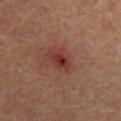{"biopsy_status": "not biopsied; imaged during a skin examination", "site": "mid back", "image": {"source": "total-body photography crop", "field_of_view_mm": 15}, "patient": {"sex": "male", "age_approx": 65}, "lesion_size": {"long_diameter_mm_approx": 3.0}, "lighting": "cross-polarized"}